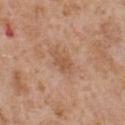Q: Is there a histopathology result?
A: total-body-photography surveillance lesion; no biopsy
Q: What lighting was used for the tile?
A: white-light
Q: What is the imaging modality?
A: total-body-photography crop, ~15 mm field of view
Q: What did automated image analysis measure?
A: an area of roughly 4 mm², an outline eccentricity of about 0.85 (0 = round, 1 = elongated), and a symmetry-axis asymmetry near 0.35; a nevus-likeness score of about 0/100 and a detector confidence of about 100 out of 100 that the crop contains a lesion
Q: What is the anatomic site?
A: the front of the torso
Q: Patient demographics?
A: male, roughly 65 years of age